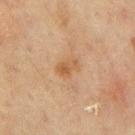{
  "biopsy_status": "not biopsied; imaged during a skin examination",
  "automated_metrics": {
    "area_mm2_approx": 4.0,
    "eccentricity": 0.75,
    "shape_asymmetry": 0.3,
    "cielab_L": 46,
    "cielab_a": 17,
    "cielab_b": 32,
    "vs_skin_darker_L": 7.0,
    "vs_skin_contrast_norm": 6.0,
    "border_irregularity_0_10": 2.5,
    "color_variation_0_10": 4.0,
    "peripheral_color_asymmetry": 1.5,
    "nevus_likeness_0_100": 5
  },
  "lesion_size": {
    "long_diameter_mm_approx": 2.5
  },
  "site": "mid back",
  "image": {
    "source": "total-body photography crop",
    "field_of_view_mm": 15
  },
  "patient": {
    "sex": "male",
    "age_approx": 70
  }
}